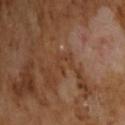Q: Was this lesion biopsied?
A: no biopsy performed (imaged during a skin exam)
Q: Automated lesion metrics?
A: a border-irregularity rating of about 8.5/10 and peripheral color asymmetry of about 0; a detector confidence of about 95 out of 100 that the crop contains a lesion
Q: What is the imaging modality?
A: 15 mm crop, total-body photography
Q: How was the tile lit?
A: cross-polarized illumination
Q: How large is the lesion?
A: ~2.5 mm (longest diameter)
Q: What are the patient's age and sex?
A: male, about 65 years old